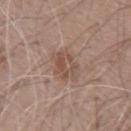  biopsy_status: not biopsied; imaged during a skin examination
  site: left upper arm
  image:
    source: total-body photography crop
    field_of_view_mm: 15
  patient:
    sex: male
    age_approx: 70